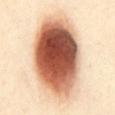A female subject aged 38 to 42. The tile uses cross-polarized illumination. Cropped from a total-body skin-imaging series; the visible field is about 15 mm. The lesion's longest dimension is about 11.5 mm. The lesion is located on the chest.